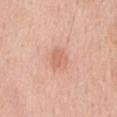biopsy status: catalogued during a skin exam; not biopsied
lesion diameter: ~2.5 mm (longest diameter)
image: ~15 mm tile from a whole-body skin photo
patient: male, about 60 years old
body site: the back
illumination: white-light